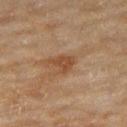This lesion was catalogued during total-body skin photography and was not selected for biopsy. The subject is a female in their 60s. Located on the right thigh. A region of skin cropped from a whole-body photographic capture, roughly 15 mm wide. Imaged with cross-polarized lighting.